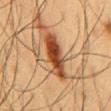Recorded during total-body skin imaging; not selected for excision or biopsy. On the front of the torso. About 6 mm across. The tile uses cross-polarized illumination. A male patient approximately 60 years of age. A lesion tile, about 15 mm wide, cut from a 3D total-body photograph.